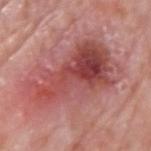Assessment: Captured during whole-body skin photography for melanoma surveillance; the lesion was not biopsied. Context: A male subject aged 73–77. The total-body-photography lesion software estimated an area of roughly 41 mm², a shape eccentricity near 0.9, and a shape-asymmetry score of about 0.4 (0 = symmetric). The software also gave a mean CIELAB color near L≈49 a*≈30 b*≈24, a lesion–skin lightness drop of about 12, and a lesion-to-skin contrast of about 8 (normalized; higher = more distinct). And it measured a nevus-likeness score of about 5/100 and a detector confidence of about 100 out of 100 that the crop contains a lesion. On the left upper arm. The tile uses white-light illumination. About 10.5 mm across. A close-up tile cropped from a whole-body skin photograph, about 15 mm across.